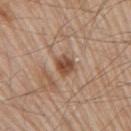Findings:
- follow-up · catalogued during a skin exam; not biopsied
- anatomic site · the right upper arm
- acquisition · 15 mm crop, total-body photography
- TBP lesion metrics · an area of roughly 5 mm²; a border-irregularity index near 2/10 and internal color variation of about 4 on a 0–10 scale
- subject · male, roughly 80 years of age
- size · ~2.5 mm (longest diameter)
- lighting · white-light